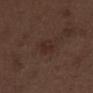Q: Is there a histopathology result?
A: imaged on a skin check; not biopsied
Q: How was this image acquired?
A: ~15 mm crop, total-body skin-cancer survey
Q: What is the anatomic site?
A: the abdomen
Q: Patient demographics?
A: male, aged 68–72
Q: Illumination type?
A: white-light